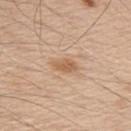biopsy_status: not biopsied; imaged during a skin examination
lesion_size:
  long_diameter_mm_approx: 3.0
patient:
  sex: male
  age_approx: 50
image:
  source: total-body photography crop
  field_of_view_mm: 15
site: upper back
lighting: white-light
automated_metrics:
  border_irregularity_0_10: 2.0
  color_variation_0_10: 2.0
  peripheral_color_asymmetry: 0.5